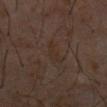{
  "biopsy_status": "not biopsied; imaged during a skin examination",
  "site": "abdomen",
  "image": {
    "source": "total-body photography crop",
    "field_of_view_mm": 15
  },
  "patient": {
    "sex": "male",
    "age_approx": 60
  }
}